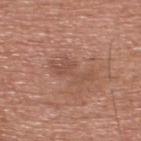imaging modality = ~15 mm tile from a whole-body skin photo | patient = male, aged approximately 55 | location = the upper back.